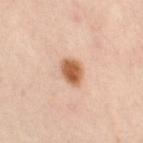| key | value |
|---|---|
| workup | catalogued during a skin exam; not biopsied |
| automated lesion analysis | internal color variation of about 4 on a 0–10 scale and radial color variation of about 1; an automated nevus-likeness rating near 100 out of 100 |
| body site | the left leg |
| lesion size | ~3.5 mm (longest diameter) |
| imaging modality | ~15 mm crop, total-body skin-cancer survey |
| subject | female, roughly 40 years of age |